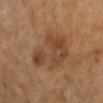Captured during whole-body skin photography for melanoma surveillance; the lesion was not biopsied. The patient is approximately 60 years of age. The lesion is on the right forearm. This image is a 15 mm lesion crop taken from a total-body photograph. About 6.5 mm across. The lesion-visualizer software estimated a footprint of about 20 mm², a shape eccentricity near 0.75, and a shape-asymmetry score of about 0.3 (0 = symmetric). The analysis additionally found an average lesion color of about L≈43 a*≈20 b*≈32 (CIELAB), a lesion–skin lightness drop of about 8, and a normalized lesion–skin contrast near 6.5. The analysis additionally found a nevus-likeness score of about 10/100 and a lesion-detection confidence of about 100/100. Imaged with cross-polarized lighting.